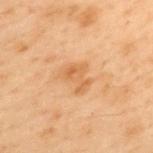The lesion was photographed on a routine skin check and not biopsied; there is no pathology result. A 15 mm crop from a total-body photograph taken for skin-cancer surveillance. This is a cross-polarized tile. Approximately 3.5 mm at its widest. Located on the mid back. A male patient, about 55 years old.